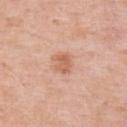Part of a total-body skin-imaging series; this lesion was reviewed on a skin check and was not flagged for biopsy.